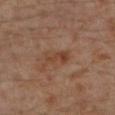{
  "biopsy_status": "not biopsied; imaged during a skin examination",
  "patient": {
    "sex": "male",
    "age_approx": 30
  },
  "site": "left lower leg",
  "image": {
    "source": "total-body photography crop",
    "field_of_view_mm": 15
  },
  "automated_metrics": {
    "area_mm2_approx": 4.5,
    "eccentricity": 0.9,
    "shape_asymmetry": 0.35,
    "cielab_L": 40,
    "cielab_a": 20,
    "cielab_b": 29,
    "vs_skin_darker_L": 7.0,
    "vs_skin_contrast_norm": 6.5,
    "border_irregularity_0_10": 4.0,
    "color_variation_0_10": 2.0,
    "peripheral_color_asymmetry": 0.5,
    "nevus_likeness_0_100": 0,
    "lesion_detection_confidence_0_100": 100
  },
  "lighting": "cross-polarized",
  "lesion_size": {
    "long_diameter_mm_approx": 3.5
  }
}